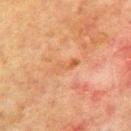Clinical summary: The patient is a male approximately 70 years of age. This is a cross-polarized tile. The recorded lesion diameter is about 3.5 mm. The lesion is located on the mid back. A 15 mm close-up tile from a total-body photography series done for melanoma screening.